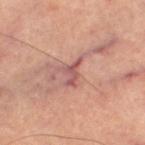Imaged during a routine full-body skin examination; the lesion was not biopsied and no histopathology is available. The lesion is on the right thigh. A male patient aged approximately 65. Cropped from a total-body skin-imaging series; the visible field is about 15 mm.